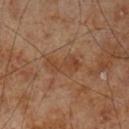notes=imaged on a skin check; not biopsied
image source=~15 mm tile from a whole-body skin photo
size=about 4.5 mm
patient=male, about 70 years old
anatomic site=the leg